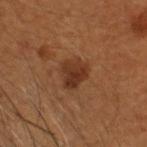Clinical impression: No biopsy was performed on this lesion — it was imaged during a full skin examination and was not determined to be concerning. Acquisition and patient details: Captured under cross-polarized illumination. The recorded lesion diameter is about 3.5 mm. Cropped from a total-body skin-imaging series; the visible field is about 15 mm. The lesion is on the head or neck. A male patient roughly 50 years of age. An algorithmic analysis of the crop reported a footprint of about 7.5 mm², an eccentricity of roughly 0.6, and a shape-asymmetry score of about 0.25 (0 = symmetric). And it measured an average lesion color of about L≈26 a*≈18 b*≈25 (CIELAB) and roughly 7 lightness units darker than nearby skin.